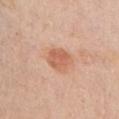Case summary:
• follow-up: imaged on a skin check; not biopsied
• site: the chest
• tile lighting: white-light
• subject: male, in their mid-70s
• imaging modality: ~15 mm crop, total-body skin-cancer survey